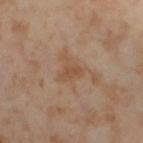Q: Where on the body is the lesion?
A: the leg
Q: What is the imaging modality?
A: 15 mm crop, total-body photography
Q: Patient demographics?
A: female, approximately 55 years of age
Q: How large is the lesion?
A: ~3 mm (longest diameter)
Q: Illumination type?
A: cross-polarized illumination
Q: What did automated image analysis measure?
A: a color-variation rating of about 1/10 and a peripheral color-asymmetry measure near 0.5